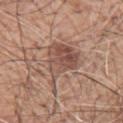Q: Where on the body is the lesion?
A: the left upper arm
Q: How was this image acquired?
A: total-body-photography crop, ~15 mm field of view
Q: What lighting was used for the tile?
A: white-light illumination
Q: Lesion size?
A: ≈7 mm
Q: What are the patient's age and sex?
A: male, roughly 60 years of age